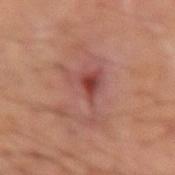notes: catalogued during a skin exam; not biopsied | acquisition: ~15 mm crop, total-body skin-cancer survey | body site: the right forearm | subject: male, approximately 55 years of age.